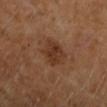Q: Was a biopsy performed?
A: imaged on a skin check; not biopsied